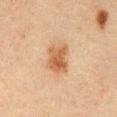A roughly 15 mm field-of-view crop from a total-body skin photograph. The tile uses cross-polarized illumination. The lesion is located on the abdomen. A male subject aged 58 to 62.